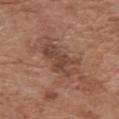Q: Was a biopsy performed?
A: total-body-photography surveillance lesion; no biopsy